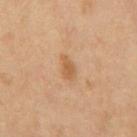Acquisition and patient details: From the back. A male subject about 55 years old. A region of skin cropped from a whole-body photographic capture, roughly 15 mm wide.